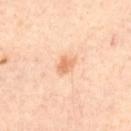Assessment: Recorded during total-body skin imaging; not selected for excision or biopsy. Acquisition and patient details: A patient aged 53 to 57. The recorded lesion diameter is about 2.5 mm. Located on the upper back. Imaged with cross-polarized lighting. A region of skin cropped from a whole-body photographic capture, roughly 15 mm wide.